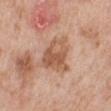  biopsy_status: not biopsied; imaged during a skin examination
  lesion_size:
    long_diameter_mm_approx: 4.0
  patient:
    sex: male
    age_approx: 80
  image:
    source: total-body photography crop
    field_of_view_mm: 15
  site: left lower leg
  lighting: white-light